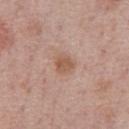Q: Was this lesion biopsied?
A: total-body-photography surveillance lesion; no biopsy